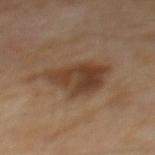| field | value |
|---|---|
| notes | no biopsy performed (imaged during a skin exam) |
| lighting | cross-polarized illumination |
| subject | male, aged 53–57 |
| lesion size | ~7 mm (longest diameter) |
| site | the mid back |
| image source | ~15 mm tile from a whole-body skin photo |